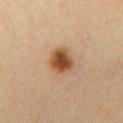Case summary:
* notes — catalogued during a skin exam; not biopsied
* imaging modality — 15 mm crop, total-body photography
* lesion diameter — ≈3.5 mm
* patient — male, about 20 years old
* tile lighting — cross-polarized illumination
* image-analysis metrics — a shape eccentricity near 0.55 and two-axis asymmetry of about 0.2; a mean CIELAB color near L≈47 a*≈21 b*≈34, a lesion–skin lightness drop of about 15, and a lesion-to-skin contrast of about 11 (normalized; higher = more distinct); a nevus-likeness score of about 100/100 and lesion-presence confidence of about 100/100
* location — the chest